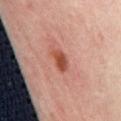Impression:
The lesion was photographed on a routine skin check and not biopsied; there is no pathology result.
Acquisition and patient details:
Measured at roughly 3.5 mm in maximum diameter. The tile uses cross-polarized illumination. This image is a 15 mm lesion crop taken from a total-body photograph. A subject in their mid-50s. On the abdomen.